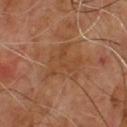Clinical impression: Captured during whole-body skin photography for melanoma surveillance; the lesion was not biopsied. Acquisition and patient details: Automated tile analysis of the lesion measured a footprint of about 16 mm², an outline eccentricity of about 0.5 (0 = round, 1 = elongated), and two-axis asymmetry of about 0.45. The analysis additionally found a lesion color around L≈44 a*≈21 b*≈32 in CIELAB, roughly 5 lightness units darker than nearby skin, and a normalized lesion–skin contrast near 4.5. It also reported a color-variation rating of about 3/10 and peripheral color asymmetry of about 1. Measured at roughly 5.5 mm in maximum diameter. The patient is a male approximately 65 years of age. A 15 mm close-up extracted from a 3D total-body photography capture. The tile uses cross-polarized illumination.